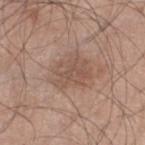Clinical impression: Part of a total-body skin-imaging series; this lesion was reviewed on a skin check and was not flagged for biopsy. Background: From the left lower leg. The tile uses white-light illumination. Approximately 4.5 mm at its widest. A 15 mm close-up tile from a total-body photography series done for melanoma screening. The patient is a male aged around 45.